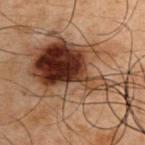Impression:
The lesion was photographed on a routine skin check and not biopsied; there is no pathology result.
Acquisition and patient details:
From the right upper arm. Longest diameter approximately 14 mm. Cropped from a total-body skin-imaging series; the visible field is about 15 mm. A male subject, aged approximately 50.